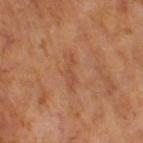A roughly 15 mm field-of-view crop from a total-body skin photograph.
About 3.5 mm across.
A male subject, aged 63 to 67.